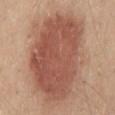Clinical impression: Part of a total-body skin-imaging series; this lesion was reviewed on a skin check and was not flagged for biopsy. Image and clinical context: Cropped from a total-body skin-imaging series; the visible field is about 15 mm. About 13 mm across. Located on the mid back. The patient is a female approximately 45 years of age.